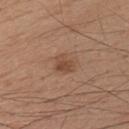<lesion>
  <biopsy_status>not biopsied; imaged during a skin examination</biopsy_status>
  <site>chest</site>
  <patient>
    <sex>male</sex>
    <age_approx>20</age_approx>
  </patient>
  <lesion_size>
    <long_diameter_mm_approx>2.5</long_diameter_mm_approx>
  </lesion_size>
  <lighting>white-light</lighting>
  <image>
    <source>total-body photography crop</source>
    <field_of_view_mm>15</field_of_view_mm>
  </image>
</lesion>